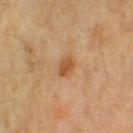Assessment:
Part of a total-body skin-imaging series; this lesion was reviewed on a skin check and was not flagged for biopsy.
Context:
The lesion is on the left thigh. An algorithmic analysis of the crop reported a lesion area of about 3.5 mm², an eccentricity of roughly 0.7, and two-axis asymmetry of about 0.25. The analysis additionally found a border-irregularity rating of about 2/10, a color-variation rating of about 2.5/10, and radial color variation of about 1. And it measured an automated nevus-likeness rating near 75 out of 100 and a lesion-detection confidence of about 100/100. A female subject, roughly 55 years of age. A lesion tile, about 15 mm wide, cut from a 3D total-body photograph.